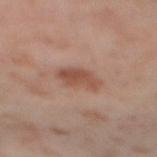| key | value |
|---|---|
| notes | catalogued during a skin exam; not biopsied |
| site | the right thigh |
| size | about 4 mm |
| acquisition | total-body-photography crop, ~15 mm field of view |
| lighting | cross-polarized illumination |
| patient | female, approximately 55 years of age |